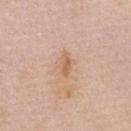Recorded during total-body skin imaging; not selected for excision or biopsy.
A female subject, approximately 65 years of age.
The total-body-photography lesion software estimated a shape-asymmetry score of about 0.25 (0 = symmetric). The software also gave a lesion–skin lightness drop of about 8 and a normalized lesion–skin contrast near 6.5. The analysis additionally found a border-irregularity index near 3/10, internal color variation of about 1 on a 0–10 scale, and a peripheral color-asymmetry measure near 0.5.
Located on the abdomen.
Cropped from a whole-body photographic skin survey; the tile spans about 15 mm.
About 3 mm across.